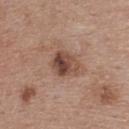The lesion was photographed on a routine skin check and not biopsied; there is no pathology result. On the upper back. Cropped from a whole-body photographic skin survey; the tile spans about 15 mm. The total-body-photography lesion software estimated an average lesion color of about L≈46 a*≈19 b*≈26 (CIELAB) and a normalized border contrast of about 9. The patient is a female in their 40s. About 4 mm across. Imaged with white-light lighting.